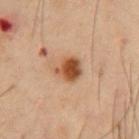{"biopsy_status": "not biopsied; imaged during a skin examination", "image": {"source": "total-body photography crop", "field_of_view_mm": 15}, "patient": {"sex": "male", "age_approx": 55}, "lesion_size": {"long_diameter_mm_approx": 3.0}, "site": "chest"}